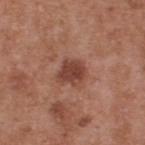Automated image analysis of the tile measured an area of roughly 7.5 mm² and a shape-asymmetry score of about 0.25 (0 = symmetric). The analysis additionally found an average lesion color of about L≈43 a*≈24 b*≈27 (CIELAB), about 11 CIELAB-L* units darker than the surrounding skin, and a lesion-to-skin contrast of about 8.5 (normalized; higher = more distinct). The software also gave a peripheral color-asymmetry measure near 1.
The lesion's longest dimension is about 3 mm.
Imaged with white-light lighting.
On the upper back.
A roughly 15 mm field-of-view crop from a total-body skin photograph.
The subject is a male aged approximately 55.